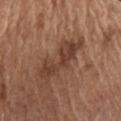A male patient, aged 73 to 77. The lesion is on the chest. A lesion tile, about 15 mm wide, cut from a 3D total-body photograph. This is a white-light tile.